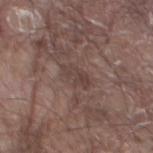biopsy status: imaged on a skin check; not biopsied | patient: male, about 80 years old | body site: the left thigh | image source: total-body-photography crop, ~15 mm field of view | illumination: white-light illumination.